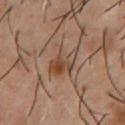biopsy_status: not biopsied; imaged during a skin examination
site: front of the torso
image:
  source: total-body photography crop
  field_of_view_mm: 15
lesion_size:
  long_diameter_mm_approx: 4.0
patient:
  sex: male
  age_approx: 55
lighting: cross-polarized